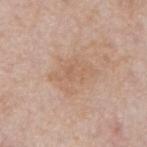Assessment:
This lesion was catalogued during total-body skin photography and was not selected for biopsy.
Context:
Located on the back. Imaged with white-light lighting. This image is a 15 mm lesion crop taken from a total-body photograph. A male patient approximately 75 years of age.